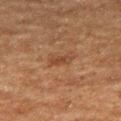Q: Was this lesion biopsied?
A: imaged on a skin check; not biopsied
Q: Illumination type?
A: cross-polarized
Q: Lesion location?
A: the right thigh
Q: What is the imaging modality?
A: total-body-photography crop, ~15 mm field of view
Q: How large is the lesion?
A: ≈3 mm
Q: Patient demographics?
A: male, approximately 75 years of age